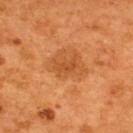Captured during whole-body skin photography for melanoma surveillance; the lesion was not biopsied. From the upper back. A male subject aged 53–57. This image is a 15 mm lesion crop taken from a total-body photograph.